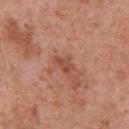Assessment:
The lesion was tiled from a total-body skin photograph and was not biopsied.